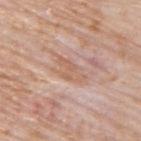{
  "site": "upper back",
  "lighting": "white-light",
  "image": {
    "source": "total-body photography crop",
    "field_of_view_mm": 15
  },
  "patient": {
    "sex": "male",
    "age_approx": 75
  }
}